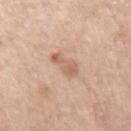Captured during whole-body skin photography for melanoma surveillance; the lesion was not biopsied.
From the right upper arm.
A roughly 15 mm field-of-view crop from a total-body skin photograph.
Imaged with white-light lighting.
Approximately 3.5 mm at its widest.
A male subject aged around 55.